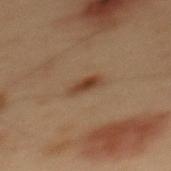The lesion was tiled from a total-body skin photograph and was not biopsied. The lesion is located on the mid back. A 15 mm close-up tile from a total-body photography series done for melanoma screening. Approximately 2.5 mm at its widest. An algorithmic analysis of the crop reported an area of roughly 3.5 mm² and a symmetry-axis asymmetry near 0.2. And it measured a mean CIELAB color near L≈33 a*≈16 b*≈26, roughly 8 lightness units darker than nearby skin, and a normalized lesion–skin contrast near 8. The analysis additionally found a classifier nevus-likeness of about 100/100 and a lesion-detection confidence of about 100/100. The tile uses cross-polarized illumination. The subject is a male aged approximately 55.